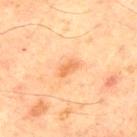image-analysis metrics: an area of roughly 3.5 mm², an outline eccentricity of about 0.85 (0 = round, 1 = elongated), and a shape-asymmetry score of about 0.4 (0 = symmetric); a lesion color around L≈56 a*≈23 b*≈37 in CIELAB, a lesion–skin lightness drop of about 8, and a normalized border contrast of about 6 | image source: ~15 mm tile from a whole-body skin photo | diameter: ≈2.5 mm | anatomic site: the chest | subject: male, approximately 65 years of age | illumination: cross-polarized.